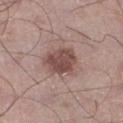Impression: The lesion was photographed on a routine skin check and not biopsied; there is no pathology result. Image and clinical context: Automated tile analysis of the lesion measured a footprint of about 11 mm², a shape eccentricity near 0.75, and a symmetry-axis asymmetry near 0.2. And it measured a nevus-likeness score of about 45/100 and a lesion-detection confidence of about 100/100. Cropped from a total-body skin-imaging series; the visible field is about 15 mm. About 4.5 mm across. This is a white-light tile. A male patient in their 50s. From the left lower leg.